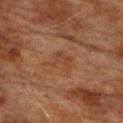Case summary:
* workup · catalogued during a skin exam; not biopsied
* image source · total-body-photography crop, ~15 mm field of view
* patient · male, approximately 75 years of age
* site · the front of the torso
* diameter · ~3.5 mm (longest diameter)
* tile lighting · cross-polarized